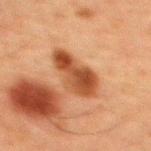Q: Is there a histopathology result?
A: no biopsy performed (imaged during a skin exam)
Q: What kind of image is this?
A: ~15 mm crop, total-body skin-cancer survey
Q: How large is the lesion?
A: about 5.5 mm
Q: What are the patient's age and sex?
A: male, aged 58–62
Q: Where on the body is the lesion?
A: the upper back